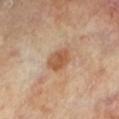follow-up = imaged on a skin check; not biopsied
lesion diameter = about 3.5 mm
image-analysis metrics = a footprint of about 6.5 mm², an eccentricity of roughly 0.75, and two-axis asymmetry of about 0.25; a mean CIELAB color near L≈52 a*≈20 b*≈33, roughly 10 lightness units darker than nearby skin, and a normalized lesion–skin contrast near 8; an automated nevus-likeness rating near 90 out of 100 and a lesion-detection confidence of about 100/100
image source = total-body-photography crop, ~15 mm field of view
lighting = cross-polarized illumination
patient = male, aged 63–67
anatomic site = the leg